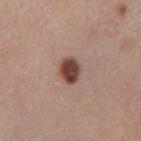The lesion-visualizer software estimated an area of roughly 6 mm² and an eccentricity of roughly 0.65. And it measured a lesion color around L≈44 a*≈20 b*≈24 in CIELAB, roughly 17 lightness units darker than nearby skin, and a lesion-to-skin contrast of about 12.5 (normalized; higher = more distinct). The analysis additionally found a classifier nevus-likeness of about 100/100 and lesion-presence confidence of about 100/100. A roughly 15 mm field-of-view crop from a total-body skin photograph. The lesion is on the abdomen. Longest diameter approximately 3 mm. A male patient aged 53–57. This is a white-light tile.